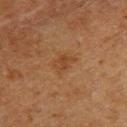Findings:
– notes — total-body-photography surveillance lesion; no biopsy
– image source — ~15 mm crop, total-body skin-cancer survey
– illumination — cross-polarized illumination
– patient — female, about 50 years old
– lesion diameter — ≈2.5 mm
– site — the upper back